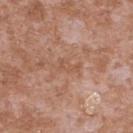Imaged during a routine full-body skin examination; the lesion was not biopsied and no histopathology is available. A 15 mm crop from a total-body photograph taken for skin-cancer surveillance. Automated image analysis of the tile measured a lesion area of about 4.5 mm², an eccentricity of roughly 0.9, and two-axis asymmetry of about 0.4. It also reported a lesion color around L≈54 a*≈21 b*≈30 in CIELAB, a lesion–skin lightness drop of about 6, and a lesion-to-skin contrast of about 5 (normalized; higher = more distinct). On the upper back. The tile uses white-light illumination. A male patient roughly 45 years of age.